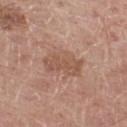| key | value |
|---|---|
| notes | imaged on a skin check; not biopsied |